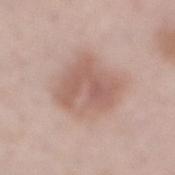{
  "biopsy_status": "not biopsied; imaged during a skin examination",
  "image": {
    "source": "total-body photography crop",
    "field_of_view_mm": 15
  },
  "patient": {
    "sex": "male",
    "age_approx": 55
  },
  "lesion_size": {
    "long_diameter_mm_approx": 5.5
  },
  "site": "mid back",
  "lighting": "white-light",
  "automated_metrics": {
    "border_irregularity_0_10": 3.0,
    "color_variation_0_10": 4.5,
    "peripheral_color_asymmetry": 1.5,
    "nevus_likeness_0_100": 10
  }
}